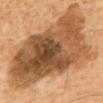workup: total-body-photography surveillance lesion; no biopsy
tile lighting: cross-polarized
subject: male, approximately 60 years of age
acquisition: 15 mm crop, total-body photography
diameter: about 18 mm
anatomic site: the chest